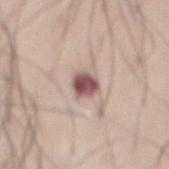Q: Is there a histopathology result?
A: catalogued during a skin exam; not biopsied
Q: What kind of image is this?
A: ~15 mm crop, total-body skin-cancer survey
Q: Who is the patient?
A: male, approximately 70 years of age
Q: What is the anatomic site?
A: the front of the torso